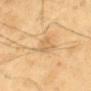workup = catalogued during a skin exam; not biopsied | image source = total-body-photography crop, ~15 mm field of view | patient = male, aged approximately 55 | site = the chest.